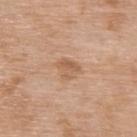<lesion>
<biopsy_status>not biopsied; imaged during a skin examination</biopsy_status>
</lesion>